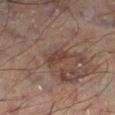Impression: The lesion was tiled from a total-body skin photograph and was not biopsied. Image and clinical context: A male patient aged approximately 55. On the left lower leg. The tile uses cross-polarized illumination. A close-up tile cropped from a whole-body skin photograph, about 15 mm across.